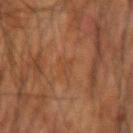acquisition: ~15 mm tile from a whole-body skin photo | diameter: ~3 mm (longest diameter) | automated lesion analysis: a footprint of about 3 mm² | location: the right forearm | subject: male, aged approximately 60.